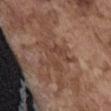patient = male, in their mid- to late 70s
illumination = white-light
lesion diameter = ~4.5 mm (longest diameter)
image = total-body-photography crop, ~15 mm field of view
location = the abdomen